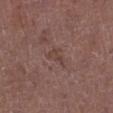follow-up: imaged on a skin check; not biopsied
tile lighting: white-light
TBP lesion metrics: a lesion area of about 3.5 mm², an outline eccentricity of about 0.75 (0 = round, 1 = elongated), and a symmetry-axis asymmetry near 0.5; a mean CIELAB color near L≈41 a*≈19 b*≈22 and a normalized lesion–skin contrast near 5
imaging modality: total-body-photography crop, ~15 mm field of view
anatomic site: the leg
patient: male, in their mid- to late 70s